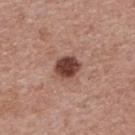image source — ~15 mm crop, total-body skin-cancer survey | tile lighting — white-light illumination | patient — female, roughly 65 years of age | body site — the back.